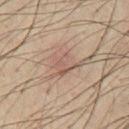patient = male, about 50 years old | site = the chest | image source = 15 mm crop, total-body photography | image-analysis metrics = an area of roughly 7 mm², a shape eccentricity near 0.7, and a symmetry-axis asymmetry near 0.4; a border-irregularity rating of about 6/10, a color-variation rating of about 3/10, and a peripheral color-asymmetry measure near 1.